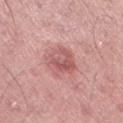Findings:
– follow-up: total-body-photography surveillance lesion; no biopsy
– lesion diameter: ≈4 mm
– subject: male, aged around 45
– image source: total-body-photography crop, ~15 mm field of view
– location: the left thigh